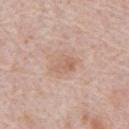Assessment:
This lesion was catalogued during total-body skin photography and was not selected for biopsy.
Context:
A male patient aged 68 to 72. Imaged with white-light lighting. The lesion is located on the abdomen. A region of skin cropped from a whole-body photographic capture, roughly 15 mm wide. The recorded lesion diameter is about 3.5 mm.